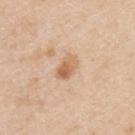• follow-up · total-body-photography surveillance lesion; no biopsy
• subject · male, in their mid- to late 40s
• imaging modality · ~15 mm tile from a whole-body skin photo
• anatomic site · the upper back